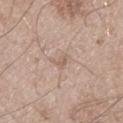This lesion was catalogued during total-body skin photography and was not selected for biopsy. The patient is a male about 65 years old. A 15 mm close-up extracted from a 3D total-body photography capture. The lesion is located on the left thigh.